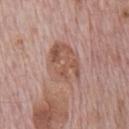workup: imaged on a skin check; not biopsied | size: about 5.5 mm | image: ~15 mm crop, total-body skin-cancer survey | image-analysis metrics: a lesion area of about 17 mm², an outline eccentricity of about 0.65 (0 = round, 1 = elongated), and a symmetry-axis asymmetry near 0.15; border irregularity of about 2 on a 0–10 scale and peripheral color asymmetry of about 2 | site: the chest | subject: male, aged around 70.